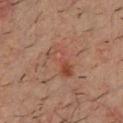Recorded during total-body skin imaging; not selected for excision or biopsy. An algorithmic analysis of the crop reported border irregularity of about 4 on a 0–10 scale, a within-lesion color-variation index near 9/10, and a peripheral color-asymmetry measure near 3. And it measured a classifier nevus-likeness of about 10/100 and lesion-presence confidence of about 100/100. Located on the chest. The subject is a male roughly 35 years of age. Imaged with cross-polarized lighting. A region of skin cropped from a whole-body photographic capture, roughly 15 mm wide.